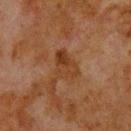Q: Was this lesion biopsied?
A: no biopsy performed (imaged during a skin exam)
Q: Lesion size?
A: about 4 mm
Q: How was this image acquired?
A: total-body-photography crop, ~15 mm field of view
Q: What lighting was used for the tile?
A: cross-polarized illumination
Q: What is the anatomic site?
A: the upper back
Q: Patient demographics?
A: male, aged approximately 80